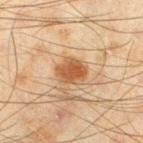Clinical impression: The lesion was tiled from a total-body skin photograph and was not biopsied. Image and clinical context: A roughly 15 mm field-of-view crop from a total-body skin photograph. Automated image analysis of the tile measured a lesion area of about 7.5 mm² and an eccentricity of roughly 0.6. The analysis additionally found a lesion color around L≈45 a*≈19 b*≈33 in CIELAB, roughly 11 lightness units darker than nearby skin, and a normalized lesion–skin contrast near 9. The tile uses cross-polarized illumination. The lesion is located on the left thigh. The subject is a male about 45 years old. The lesion's longest dimension is about 3.5 mm.